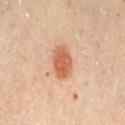Captured during whole-body skin photography for melanoma surveillance; the lesion was not biopsied.